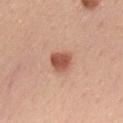Case summary:
– biopsy status: total-body-photography surveillance lesion; no biopsy
– illumination: white-light illumination
– subject: male, roughly 60 years of age
– body site: the upper back
– image source: total-body-photography crop, ~15 mm field of view
– size: ~3 mm (longest diameter)
– automated lesion analysis: an average lesion color of about L≈54 a*≈26 b*≈30 (CIELAB), roughly 14 lightness units darker than nearby skin, and a lesion-to-skin contrast of about 9.5 (normalized; higher = more distinct); a border-irregularity rating of about 2/10, a color-variation rating of about 3/10, and radial color variation of about 1; a classifier nevus-likeness of about 100/100 and lesion-presence confidence of about 100/100